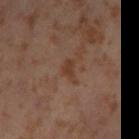Assessment: Captured during whole-body skin photography for melanoma surveillance; the lesion was not biopsied. Image and clinical context: Cropped from a whole-body photographic skin survey; the tile spans about 15 mm. This is a cross-polarized tile. The lesion is on the left thigh. The lesion-visualizer software estimated a lesion area of about 3.5 mm² and a shape eccentricity near 0.85. Measured at roughly 3 mm in maximum diameter. A female subject approximately 55 years of age.